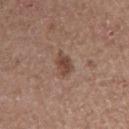illumination: white-light illumination
patient: male, about 60 years old
lesion size: about 3 mm
anatomic site: the arm
image source: 15 mm crop, total-body photography